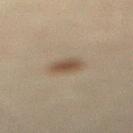<record>
  <site>right lower leg</site>
  <image>
    <source>total-body photography crop</source>
    <field_of_view_mm>15</field_of_view_mm>
  </image>
  <lighting>cross-polarized</lighting>
  <lesion_size>
    <long_diameter_mm_approx>3.0</long_diameter_mm_approx>
  </lesion_size>
  <patient>
    <sex>female</sex>
    <age_approx>65</age_approx>
  </patient>
  <automated_metrics>
    <area_mm2_approx>5.0</area_mm2_approx>
    <eccentricity>0.7</eccentricity>
    <shape_asymmetry>0.2</shape_asymmetry>
    <border_irregularity_0_10>2.0</border_irregularity_0_10>
    <peripheral_color_asymmetry>1.0</peripheral_color_asymmetry>
    <lesion_detection_confidence_0_100>100</lesion_detection_confidence_0_100>
  </automated_metrics>
</record>